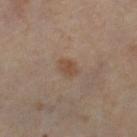Q: What kind of image is this?
A: 15 mm crop, total-body photography
Q: What is the lesion's diameter?
A: about 2.5 mm
Q: Illumination type?
A: cross-polarized
Q: Where on the body is the lesion?
A: the left thigh
Q: What did automated image analysis measure?
A: lesion-presence confidence of about 100/100
Q: Patient demographics?
A: female, aged 48–52